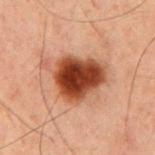Part of a total-body skin-imaging series; this lesion was reviewed on a skin check and was not flagged for biopsy. A lesion tile, about 15 mm wide, cut from a 3D total-body photograph. A male subject, approximately 60 years of age. Located on the mid back. Automated image analysis of the tile measured a footprint of about 22 mm² and a shape-asymmetry score of about 0.3 (0 = symmetric). It also reported an average lesion color of about L≈35 a*≈22 b*≈28 (CIELAB), a lesion–skin lightness drop of about 16, and a normalized lesion–skin contrast near 13.5. It also reported border irregularity of about 4 on a 0–10 scale, internal color variation of about 7 on a 0–10 scale, and a peripheral color-asymmetry measure near 2. The recorded lesion diameter is about 7 mm.